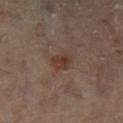Captured during whole-body skin photography for melanoma surveillance; the lesion was not biopsied.
A female subject aged 58–62.
A 15 mm close-up tile from a total-body photography series done for melanoma screening.
The total-body-photography lesion software estimated a footprint of about 4 mm², an eccentricity of roughly 0.65, and two-axis asymmetry of about 0.35. It also reported a classifier nevus-likeness of about 85/100.
The lesion is on the leg.
Imaged with cross-polarized lighting.